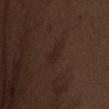follow-up: total-body-photography surveillance lesion; no biopsy
lighting: white-light illumination
lesion size: ≈3.5 mm
automated lesion analysis: a border-irregularity index near 3/10 and a color-variation rating of about 1/10
imaging modality: ~15 mm tile from a whole-body skin photo
subject: male, aged approximately 70
body site: the chest